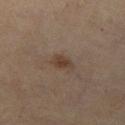Imaged during a routine full-body skin examination; the lesion was not biopsied and no histopathology is available.